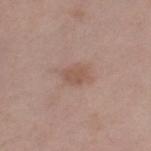Assessment: No biopsy was performed on this lesion — it was imaged during a full skin examination and was not determined to be concerning. Clinical summary: A region of skin cropped from a whole-body photographic capture, roughly 15 mm wide. The lesion is on the left thigh. The tile uses white-light illumination. About 2.5 mm across. A female subject aged 63 to 67. Automated image analysis of the tile measured an average lesion color of about L≈53 a*≈19 b*≈26 (CIELAB), about 7 CIELAB-L* units darker than the surrounding skin, and a normalized lesion–skin contrast near 6. The analysis additionally found a border-irregularity rating of about 2/10, a within-lesion color-variation index near 1.5/10, and radial color variation of about 0.5. And it measured lesion-presence confidence of about 100/100.